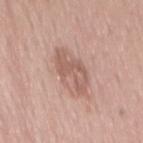Findings:
* follow-up — no biopsy performed (imaged during a skin exam)
* lesion diameter — about 5.5 mm
* body site — the mid back
* patient — female, in their mid- to late 50s
* lighting — white-light illumination
* imaging modality — ~15 mm tile from a whole-body skin photo